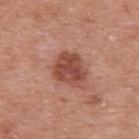The lesion was photographed on a routine skin check and not biopsied; there is no pathology result.
A male subject, roughly 50 years of age.
A region of skin cropped from a whole-body photographic capture, roughly 15 mm wide.
Located on the upper back.